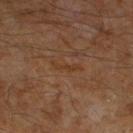Measured at roughly 3.5 mm in maximum diameter. Automated image analysis of the tile measured a footprint of about 2.5 mm² and two-axis asymmetry of about 0.55. It also reported about 4 CIELAB-L* units darker than the surrounding skin. The software also gave a border-irregularity rating of about 6.5/10, a within-lesion color-variation index near 0/10, and a peripheral color-asymmetry measure near 0. The lesion is located on the left lower leg. A close-up tile cropped from a whole-body skin photograph, about 15 mm across. Captured under cross-polarized illumination. A male patient roughly 60 years of age.